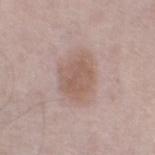Impression: No biopsy was performed on this lesion — it was imaged during a full skin examination and was not determined to be concerning. Image and clinical context: The lesion-visualizer software estimated an area of roughly 13 mm², an outline eccentricity of about 0.7 (0 = round, 1 = elongated), and a shape-asymmetry score of about 0.15 (0 = symmetric). And it measured a lesion color around L≈57 a*≈17 b*≈24 in CIELAB and a normalized border contrast of about 6.5. And it measured a border-irregularity rating of about 2/10, a within-lesion color-variation index near 2.5/10, and radial color variation of about 0.5. A region of skin cropped from a whole-body photographic capture, roughly 15 mm wide. A male patient, aged approximately 65. About 5 mm across. On the left thigh.